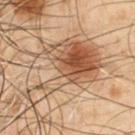The lesion was photographed on a routine skin check and not biopsied; there is no pathology result. A 15 mm crop from a total-body photograph taken for skin-cancer surveillance. An algorithmic analysis of the crop reported a lesion area of about 31 mm² and a shape-asymmetry score of about 0.6 (0 = symmetric). The analysis additionally found an average lesion color of about L≈41 a*≈15 b*≈26 (CIELAB), roughly 9 lightness units darker than nearby skin, and a lesion-to-skin contrast of about 8 (normalized; higher = more distinct). The analysis additionally found a within-lesion color-variation index near 8/10 and radial color variation of about 2.5. Located on the left upper arm. Measured at roughly 9.5 mm in maximum diameter. The tile uses cross-polarized illumination. A male patient, roughly 50 years of age.